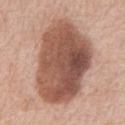Case summary:
• image source — ~15 mm crop, total-body skin-cancer survey
• location — the chest
• subject — male, aged approximately 75